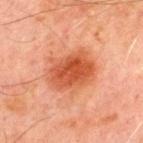Measured at roughly 5.5 mm in maximum diameter. From the chest. A roughly 15 mm field-of-view crop from a total-body skin photograph. Automated tile analysis of the lesion measured roughly 11 lightness units darker than nearby skin and a lesion-to-skin contrast of about 8.5 (normalized; higher = more distinct). The analysis additionally found border irregularity of about 2.5 on a 0–10 scale and radial color variation of about 1.5. The patient is a male roughly 70 years of age.